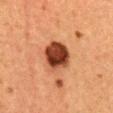{
  "biopsy_status": "not biopsied; imaged during a skin examination",
  "lesion_size": {
    "long_diameter_mm_approx": 4.0
  },
  "lighting": "cross-polarized",
  "site": "mid back",
  "automated_metrics": {
    "area_mm2_approx": 11.0,
    "shape_asymmetry": 0.15,
    "nevus_likeness_0_100": 60
  },
  "image": {
    "source": "total-body photography crop",
    "field_of_view_mm": 15
  },
  "patient": {
    "sex": "female",
    "age_approx": 40
  }
}